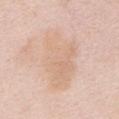follow-up: total-body-photography surveillance lesion; no biopsy
image: total-body-photography crop, ~15 mm field of view
site: the chest
diameter: ~7.5 mm (longest diameter)
subject: female, aged approximately 70